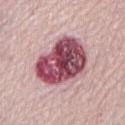* notes · catalogued during a skin exam; not biopsied
* body site · the abdomen
* illumination · white-light illumination
* subject · female, aged 63–67
* image source · total-body-photography crop, ~15 mm field of view